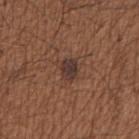• biopsy status — imaged on a skin check; not biopsied
• image — total-body-photography crop, ~15 mm field of view
• size — about 2.5 mm
• body site — the right upper arm
• patient — male, aged 63 to 67